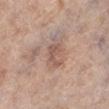notes=total-body-photography surveillance lesion; no biopsy
illumination=white-light
subject=female, approximately 65 years of age
image=15 mm crop, total-body photography
anatomic site=the left lower leg